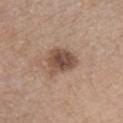This lesion was catalogued during total-body skin photography and was not selected for biopsy. A 15 mm close-up tile from a total-body photography series done for melanoma screening. The subject is a female roughly 45 years of age. On the front of the torso. Measured at roughly 4 mm in maximum diameter.